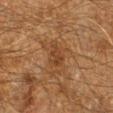• follow-up — total-body-photography surveillance lesion; no biopsy
• patient — male, aged approximately 60
• image — total-body-photography crop, ~15 mm field of view
• site — the leg